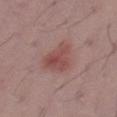Case summary:
- patient — male, roughly 40 years of age
- diameter — about 4 mm
- acquisition — ~15 mm crop, total-body skin-cancer survey
- tile lighting — white-light
- site — the right thigh